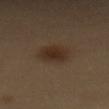Impression: The lesion was tiled from a total-body skin photograph and was not biopsied. Context: About 3.5 mm across. Imaged with cross-polarized lighting. This image is a 15 mm lesion crop taken from a total-body photograph. A female subject aged 58–62. From the abdomen.